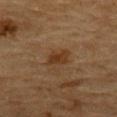• biopsy status: no biopsy performed (imaged during a skin exam)
• patient: male, aged approximately 85 or older
• tile lighting: cross-polarized illumination
• image: total-body-photography crop, ~15 mm field of view
• lesion size: about 2.5 mm
• body site: the upper back